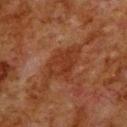imaging modality — 15 mm crop, total-body photography | subject — male, aged 78–82 | lesion diameter — about 5 mm | image-analysis metrics — a mean CIELAB color near L≈27 a*≈22 b*≈27, roughly 6 lightness units darker than nearby skin, and a normalized lesion–skin contrast near 6.5; border irregularity of about 3.5 on a 0–10 scale | tile lighting — cross-polarized illumination | location — the upper back.